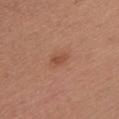<case>
  <biopsy_status>not biopsied; imaged during a skin examination</biopsy_status>
  <patient>
    <sex>female</sex>
    <age_approx>25</age_approx>
  </patient>
  <lighting>white-light</lighting>
  <lesion_size>
    <long_diameter_mm_approx>2.5</long_diameter_mm_approx>
  </lesion_size>
  <image>
    <source>total-body photography crop</source>
    <field_of_view_mm>15</field_of_view_mm>
  </image>
  <site>chest</site>
</case>